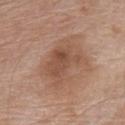Q: Was a biopsy performed?
A: imaged on a skin check; not biopsied
Q: How large is the lesion?
A: about 5 mm
Q: Who is the patient?
A: male, roughly 80 years of age
Q: Where on the body is the lesion?
A: the chest
Q: Illumination type?
A: white-light
Q: What kind of image is this?
A: ~15 mm tile from a whole-body skin photo